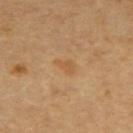The lesion was photographed on a routine skin check and not biopsied; there is no pathology result. A patient roughly 60 years of age. The lesion is located on the upper back. A roughly 15 mm field-of-view crop from a total-body skin photograph. Imaged with cross-polarized lighting.